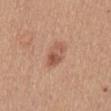workup=no biopsy performed (imaged during a skin exam); size=≈3 mm; anatomic site=the front of the torso; patient=female, aged approximately 55; image=15 mm crop, total-body photography; illumination=white-light.